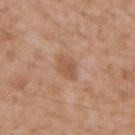Q: Was a biopsy performed?
A: total-body-photography surveillance lesion; no biopsy
Q: What kind of image is this?
A: 15 mm crop, total-body photography
Q: What are the patient's age and sex?
A: male, approximately 65 years of age
Q: Lesion location?
A: the back